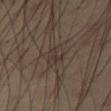Assessment:
This lesion was catalogued during total-body skin photography and was not selected for biopsy.
Acquisition and patient details:
The total-body-photography lesion software estimated a border-irregularity index near 3/10, internal color variation of about 4.5 on a 0–10 scale, and peripheral color asymmetry of about 2. It also reported lesion-presence confidence of about 70/100. A 15 mm close-up extracted from a 3D total-body photography capture. About 3.5 mm across. On the right thigh. Captured under cross-polarized illumination. A male subject, about 40 years old.